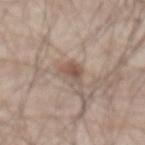Q: Was a biopsy performed?
A: imaged on a skin check; not biopsied
Q: What is the imaging modality?
A: ~15 mm crop, total-body skin-cancer survey
Q: What are the patient's age and sex?
A: male, approximately 65 years of age
Q: Lesion location?
A: the abdomen
Q: Illumination type?
A: white-light
Q: How large is the lesion?
A: about 3.5 mm